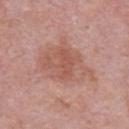TBP lesion metrics: an area of roughly 22 mm², an eccentricity of roughly 0.7, and a symmetry-axis asymmetry near 0.25; an average lesion color of about L≈56 a*≈23 b*≈27 (CIELAB) and about 8 CIELAB-L* units darker than the surrounding skin
patient: male, roughly 55 years of age
imaging modality: ~15 mm crop, total-body skin-cancer survey
location: the front of the torso